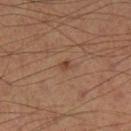This lesion was catalogued during total-body skin photography and was not selected for biopsy. The patient is a male aged approximately 55. A 15 mm crop from a total-body photograph taken for skin-cancer surveillance. From the left lower leg.